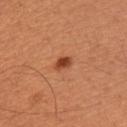This lesion was catalogued during total-body skin photography and was not selected for biopsy.
A male patient, in their mid-30s.
On the right upper arm.
Longest diameter approximately 2 mm.
A 15 mm close-up extracted from a 3D total-body photography capture.